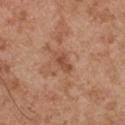workup: catalogued during a skin exam; not biopsied
acquisition: 15 mm crop, total-body photography
automated lesion analysis: an area of roughly 4 mm², an eccentricity of roughly 0.85, and a symmetry-axis asymmetry near 0.3; a mean CIELAB color near L≈50 a*≈23 b*≈32, roughly 8 lightness units darker than nearby skin, and a lesion-to-skin contrast of about 6 (normalized; higher = more distinct); a color-variation rating of about 2/10 and peripheral color asymmetry of about 0.5
subject: male, about 55 years old
body site: the left upper arm
size: ≈3 mm
illumination: white-light